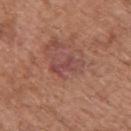– workup: catalogued during a skin exam; not biopsied
– size: ≈4 mm
– automated lesion analysis: a lesion area of about 6.5 mm², a shape eccentricity near 0.85, and two-axis asymmetry of about 0.35; a mean CIELAB color near L≈47 a*≈24 b*≈24, about 7 CIELAB-L* units darker than the surrounding skin, and a normalized border contrast of about 6.5; border irregularity of about 5 on a 0–10 scale and internal color variation of about 3 on a 0–10 scale
– subject: male, about 75 years old
– acquisition: total-body-photography crop, ~15 mm field of view
– illumination: white-light
– body site: the upper back